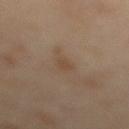Case summary:
– biopsy status: imaged on a skin check; not biopsied
– lesion diameter: ~2.5 mm (longest diameter)
– TBP lesion metrics: an outline eccentricity of about 0.85 (0 = round, 1 = elongated) and a symmetry-axis asymmetry near 0.35; a lesion color around L≈46 a*≈14 b*≈29 in CIELAB, a lesion–skin lightness drop of about 6, and a normalized border contrast of about 5.5; border irregularity of about 3 on a 0–10 scale, internal color variation of about 0.5 on a 0–10 scale, and peripheral color asymmetry of about 0; a nevus-likeness score of about 0/100
– patient: female, in their mid-50s
– location: the mid back
– acquisition: total-body-photography crop, ~15 mm field of view